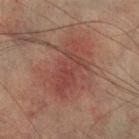Part of a total-body skin-imaging series; this lesion was reviewed on a skin check and was not flagged for biopsy. Automated tile analysis of the lesion measured a footprint of about 25 mm² and a symmetry-axis asymmetry near 0.3. It also reported about 6 CIELAB-L* units darker than the surrounding skin and a lesion-to-skin contrast of about 5.5 (normalized; higher = more distinct). It also reported a border-irregularity index near 4/10, a within-lesion color-variation index near 4.5/10, and radial color variation of about 1.5. A male patient, about 75 years old. This is a cross-polarized tile. A 15 mm close-up extracted from a 3D total-body photography capture. The lesion is located on the right lower leg.